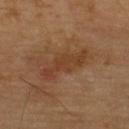Case summary:
– follow-up — imaged on a skin check; not biopsied
– diameter — about 6.5 mm
– image source — 15 mm crop, total-body photography
– site — the upper back
– tile lighting — cross-polarized illumination
– patient — male, aged approximately 70
– automated metrics — a footprint of about 10 mm², an eccentricity of roughly 0.95, and a shape-asymmetry score of about 0.3 (0 = symmetric); an average lesion color of about L≈37 a*≈20 b*≈30 (CIELAB), a lesion–skin lightness drop of about 7, and a lesion-to-skin contrast of about 6.5 (normalized; higher = more distinct); border irregularity of about 5 on a 0–10 scale and radial color variation of about 0.5